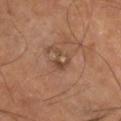Part of a total-body skin-imaging series; this lesion was reviewed on a skin check and was not flagged for biopsy.
The subject is a male aged around 65.
Cropped from a whole-body photographic skin survey; the tile spans about 15 mm.
The lesion is located on the left lower leg.